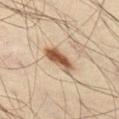Q: Was this lesion biopsied?
A: catalogued during a skin exam; not biopsied
Q: Lesion size?
A: about 4.5 mm
Q: Lesion location?
A: the left thigh
Q: What is the imaging modality?
A: 15 mm crop, total-body photography
Q: Who is the patient?
A: male, aged 48–52